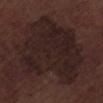Assessment:
Part of a total-body skin-imaging series; this lesion was reviewed on a skin check and was not flagged for biopsy.
Background:
The lesion is on the leg. A male patient aged 68–72. Cropped from a whole-body photographic skin survey; the tile spans about 15 mm. About 9.5 mm across. This is a white-light tile. Automated image analysis of the tile measured about 6 CIELAB-L* units darker than the surrounding skin and a normalized lesion–skin contrast near 8. And it measured a nevus-likeness score of about 0/100 and a detector confidence of about 90 out of 100 that the crop contains a lesion.